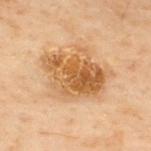Assessment: The lesion was tiled from a total-body skin photograph and was not biopsied. Clinical summary: The lesion-visualizer software estimated an outline eccentricity of about 0.55 (0 = round, 1 = elongated). It also reported roughly 10 lightness units darker than nearby skin. The analysis additionally found a border-irregularity rating of about 3.5/10 and a within-lesion color-variation index near 7/10. A lesion tile, about 15 mm wide, cut from a 3D total-body photograph. A male subject approximately 50 years of age. The lesion is located on the upper back. Imaged with cross-polarized lighting.